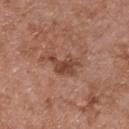The lesion was tiled from a total-body skin photograph and was not biopsied. The patient is a male aged 53 to 57. About 4 mm across. A close-up tile cropped from a whole-body skin photograph, about 15 mm across. The lesion is located on the chest. The tile uses white-light illumination.